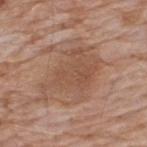lighting = white-light
location = the upper back
patient = male, roughly 60 years of age
imaging modality = 15 mm crop, total-body photography
lesion diameter = ≈7 mm
image-analysis metrics = a lesion color around L≈51 a*≈20 b*≈30 in CIELAB, about 8 CIELAB-L* units darker than the surrounding skin, and a normalized lesion–skin contrast near 6; a nevus-likeness score of about 10/100 and lesion-presence confidence of about 100/100